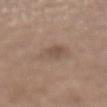{
  "biopsy_status": "not biopsied; imaged during a skin examination",
  "lighting": "white-light",
  "image": {
    "source": "total-body photography crop",
    "field_of_view_mm": 15
  },
  "lesion_size": {
    "long_diameter_mm_approx": 4.0
  },
  "patient": {
    "sex": "female",
    "age_approx": 65
  },
  "site": "abdomen"
}